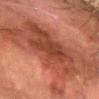Imaged during a routine full-body skin examination; the lesion was not biopsied and no histopathology is available.
A 15 mm crop from a total-body photograph taken for skin-cancer surveillance.
A male subject roughly 80 years of age.
Captured under cross-polarized illumination.
Measured at roughly 11 mm in maximum diameter.
The lesion-visualizer software estimated a border-irregularity rating of about 8/10, internal color variation of about 4.5 on a 0–10 scale, and peripheral color asymmetry of about 1.5. The software also gave a detector confidence of about 85 out of 100 that the crop contains a lesion.
From the left forearm.